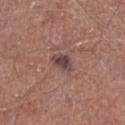follow-up: imaged on a skin check; not biopsied
image source: 15 mm crop, total-body photography
patient: male, about 70 years old
illumination: white-light illumination
anatomic site: the right lower leg